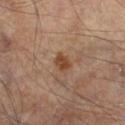* automated lesion analysis: a lesion color around L≈43 a*≈20 b*≈31 in CIELAB and a lesion-to-skin contrast of about 8.5 (normalized; higher = more distinct)
* lighting: cross-polarized
* patient: male, approximately 65 years of age
* image: 15 mm crop, total-body photography
* diameter: about 2.5 mm
* site: the right lower leg